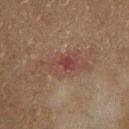tile lighting: cross-polarized illumination; acquisition: total-body-photography crop, ~15 mm field of view; location: the left lower leg; subject: male, in their mid-60s.